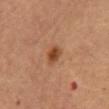Assessment: This lesion was catalogued during total-body skin photography and was not selected for biopsy.